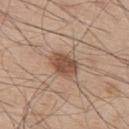{
  "biopsy_status": "not biopsied; imaged during a skin examination",
  "lighting": "white-light",
  "patient": {
    "sex": "male",
    "age_approx": 55
  },
  "image": {
    "source": "total-body photography crop",
    "field_of_view_mm": 15
  },
  "site": "upper back",
  "lesion_size": {
    "long_diameter_mm_approx": 3.5
  }
}